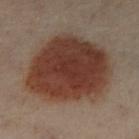{"biopsy_status": "not biopsied; imaged during a skin examination"}